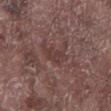No biopsy was performed on this lesion — it was imaged during a full skin examination and was not determined to be concerning. The tile uses white-light illumination. A roughly 15 mm field-of-view crop from a total-body skin photograph. The recorded lesion diameter is about 3 mm. A male subject roughly 75 years of age. The lesion is located on the left lower leg.